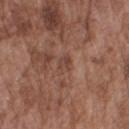| feature | finding |
|---|---|
| workup | imaged on a skin check; not biopsied |
| patient | male, aged approximately 75 |
| site | the right upper arm |
| tile lighting | white-light illumination |
| lesion diameter | ≈2.5 mm |
| image | ~15 mm tile from a whole-body skin photo |